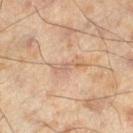Located on the left lower leg. A male patient about 45 years old. A 15 mm close-up tile from a total-body photography series done for melanoma screening. The tile uses cross-polarized illumination.